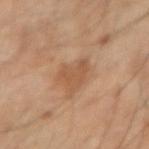biopsy status: imaged on a skin check; not biopsied | image: 15 mm crop, total-body photography | subject: male, aged around 55 | location: the right forearm | lesion size: ≈3.5 mm.